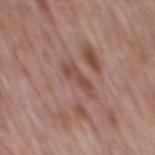* workup: no biopsy performed (imaged during a skin exam)
* lighting: white-light
* acquisition: ~15 mm tile from a whole-body skin photo
* site: the mid back
* diameter: ~3.5 mm (longest diameter)
* subject: male, roughly 70 years of age